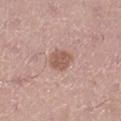Q: Was this lesion biopsied?
A: no biopsy performed (imaged during a skin exam)
Q: Patient demographics?
A: male, aged approximately 35
Q: Automated lesion metrics?
A: a border-irregularity rating of about 1.5/10 and internal color variation of about 2 on a 0–10 scale; an automated nevus-likeness rating near 45 out of 100 and lesion-presence confidence of about 100/100
Q: What lighting was used for the tile?
A: white-light
Q: What is the imaging modality?
A: ~15 mm tile from a whole-body skin photo
Q: What is the lesion's diameter?
A: ~3 mm (longest diameter)
Q: Lesion location?
A: the right lower leg